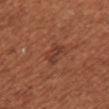workup — no biopsy performed (imaged during a skin exam)
location — the front of the torso
patient — female, aged 63–67
automated metrics — a lesion area of about 3.5 mm², a shape eccentricity near 0.85, and a symmetry-axis asymmetry near 0.3; a border-irregularity index near 3/10 and a peripheral color-asymmetry measure near 0.5
acquisition — 15 mm crop, total-body photography